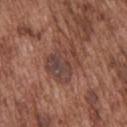Q: Was this lesion biopsied?
A: catalogued during a skin exam; not biopsied
Q: Who is the patient?
A: male, in their mid-70s
Q: Where on the body is the lesion?
A: the back
Q: Illumination type?
A: white-light illumination
Q: What is the imaging modality?
A: 15 mm crop, total-body photography
Q: What did automated image analysis measure?
A: a footprint of about 13 mm² and a symmetry-axis asymmetry near 0.4; an average lesion color of about L≈42 a*≈20 b*≈23 (CIELAB) and a normalized lesion–skin contrast near 7; an automated nevus-likeness rating near 0 out of 100